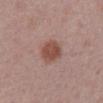Q: Was this lesion biopsied?
A: imaged on a skin check; not biopsied